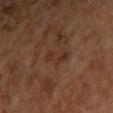workup: no biopsy performed (imaged during a skin exam) | body site: the left forearm | subject: female, aged 58 to 62 | image source: ~15 mm tile from a whole-body skin photo | automated lesion analysis: a border-irregularity index near 4.5/10 and internal color variation of about 1.5 on a 0–10 scale; an automated nevus-likeness rating near 0 out of 100 and a detector confidence of about 100 out of 100 that the crop contains a lesion | illumination: cross-polarized.